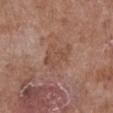workup — total-body-photography surveillance lesion; no biopsy
lesion size — about 4 mm
body site — the chest
lighting — white-light
subject — female, in their 80s
image — ~15 mm tile from a whole-body skin photo